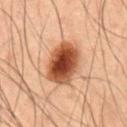| field | value |
|---|---|
| workup | total-body-photography surveillance lesion; no biopsy |
| diameter | ≈5 mm |
| body site | the abdomen |
| patient | male, about 50 years old |
| imaging modality | 15 mm crop, total-body photography |